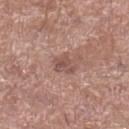This is a white-light tile. Approximately 2.5 mm at its widest. A region of skin cropped from a whole-body photographic capture, roughly 15 mm wide. A male patient in their mid-60s. The lesion-visualizer software estimated an area of roughly 4.5 mm², an eccentricity of roughly 0.6, and two-axis asymmetry of about 0.3. And it measured a classifier nevus-likeness of about 0/100 and lesion-presence confidence of about 100/100. The lesion is located on the leg.